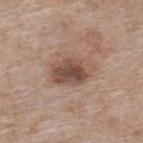  biopsy_status: not biopsied; imaged during a skin examination
  lesion_size:
    long_diameter_mm_approx: 5.0
  image:
    source: total-body photography crop
    field_of_view_mm: 15
  site: upper back
  lighting: white-light
  patient:
    sex: female
    age_approx: 75